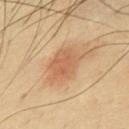{"patient": {"sex": "male", "age_approx": 40}, "lighting": "cross-polarized", "image": {"source": "total-body photography crop", "field_of_view_mm": 15}, "lesion_size": {"long_diameter_mm_approx": 5.0}, "site": "chest", "automated_metrics": {"eccentricity": 0.75, "shape_asymmetry": 0.2, "vs_skin_darker_L": 9.0, "vs_skin_contrast_norm": 6.0, "border_irregularity_0_10": 2.5, "color_variation_0_10": 3.5, "nevus_likeness_0_100": 95, "lesion_detection_confidence_0_100": 100}}